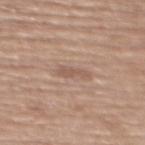Q: Is there a histopathology result?
A: no biopsy performed (imaged during a skin exam)
Q: What are the patient's age and sex?
A: female, aged approximately 70
Q: Lesion location?
A: the upper back
Q: How was this image acquired?
A: total-body-photography crop, ~15 mm field of view